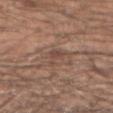The lesion was tiled from a total-body skin photograph and was not biopsied. The lesion is located on the right forearm. Imaged with white-light lighting. A 15 mm close-up extracted from a 3D total-body photography capture. Automated image analysis of the tile measured a lesion area of about 3 mm² and an eccentricity of roughly 0.85. It also reported a lesion color around L≈46 a*≈18 b*≈25 in CIELAB and roughly 7 lightness units darker than nearby skin. The software also gave an automated nevus-likeness rating near 0 out of 100 and a detector confidence of about 50 out of 100 that the crop contains a lesion. The lesion's longest dimension is about 3 mm. The subject is a male aged around 50.